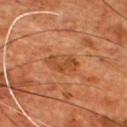Findings:
* biopsy status — imaged on a skin check; not biopsied
* patient — male, aged around 55
* body site — the chest
* acquisition — 15 mm crop, total-body photography